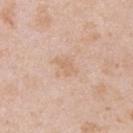Imaged during a routine full-body skin examination; the lesion was not biopsied and no histopathology is available.
The lesion is on the upper back.
Cropped from a total-body skin-imaging series; the visible field is about 15 mm.
The subject is a male in their mid-20s.
The tile uses white-light illumination.